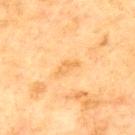<case>
  <biopsy_status>not biopsied; imaged during a skin examination</biopsy_status>
  <lesion_size>
    <long_diameter_mm_approx>2.5</long_diameter_mm_approx>
  </lesion_size>
  <patient>
    <sex>male</sex>
    <age_approx>70</age_approx>
  </patient>
  <automated_metrics>
    <nevus_likeness_0_100>0</nevus_likeness_0_100>
    <lesion_detection_confidence_0_100>100</lesion_detection_confidence_0_100>
  </automated_metrics>
  <image>
    <source>total-body photography crop</source>
    <field_of_view_mm>15</field_of_view_mm>
  </image>
  <lighting>cross-polarized</lighting>
  <site>upper back</site>
</case>